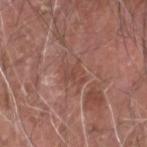notes=no biopsy performed (imaged during a skin exam)
body site=the head or neck
subject=male, roughly 75 years of age
image=total-body-photography crop, ~15 mm field of view
diameter=≈3 mm
tile lighting=white-light